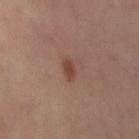No biopsy was performed on this lesion — it was imaged during a full skin examination and was not determined to be concerning.
A female subject in their 40s.
The lesion is located on the abdomen.
Cropped from a total-body skin-imaging series; the visible field is about 15 mm.
Longest diameter approximately 2.5 mm.
Automated image analysis of the tile measured an average lesion color of about L≈43 a*≈22 b*≈28 (CIELAB), a lesion–skin lightness drop of about 9, and a lesion-to-skin contrast of about 8 (normalized; higher = more distinct). The analysis additionally found an automated nevus-likeness rating near 95 out of 100 and a detector confidence of about 100 out of 100 that the crop contains a lesion.
Captured under cross-polarized illumination.